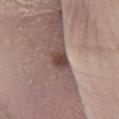No biopsy was performed on this lesion — it was imaged during a full skin examination and was not determined to be concerning. A female subject in their mid-60s. On the left lower leg. Cropped from a whole-body photographic skin survey; the tile spans about 15 mm. Imaged with white-light lighting. About 3 mm across. Automated tile analysis of the lesion measured an area of roughly 4.5 mm². The software also gave a normalized lesion–skin contrast near 10.5. The analysis additionally found a border-irregularity index near 1.5/10 and a color-variation rating of about 3/10.